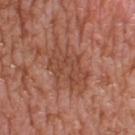workup: catalogued during a skin exam; not biopsied | anatomic site: the right thigh | patient: female, in their 40s | automated metrics: a lesion area of about 13 mm², an eccentricity of roughly 0.8, and a shape-asymmetry score of about 0.3 (0 = symmetric); border irregularity of about 5 on a 0–10 scale; a nevus-likeness score of about 0/100 and a lesion-detection confidence of about 95/100 | imaging modality: total-body-photography crop, ~15 mm field of view | size: about 5.5 mm.